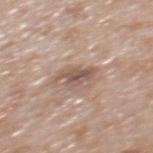<lesion>
  <biopsy_status>not biopsied; imaged during a skin examination</biopsy_status>
  <site>back</site>
  <patient>
    <sex>male</sex>
    <age_approx>65</age_approx>
  </patient>
  <lesion_size>
    <long_diameter_mm_approx>3.5</long_diameter_mm_approx>
  </lesion_size>
  <image>
    <source>total-body photography crop</source>
    <field_of_view_mm>15</field_of_view_mm>
  </image>
</lesion>